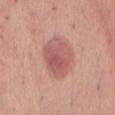Part of a total-body skin-imaging series; this lesion was reviewed on a skin check and was not flagged for biopsy.
The lesion is located on the front of the torso.
This is a white-light tile.
A male patient aged approximately 35.
Approximately 5 mm at its widest.
The lesion-visualizer software estimated an area of roughly 15 mm², an outline eccentricity of about 0.7 (0 = round, 1 = elongated), and two-axis asymmetry of about 0.1. And it measured a lesion color around L≈57 a*≈27 b*≈24 in CIELAB and a normalized lesion–skin contrast near 7. The analysis additionally found a border-irregularity index near 1/10, a within-lesion color-variation index near 4.5/10, and peripheral color asymmetry of about 1.5. The software also gave a classifier nevus-likeness of about 90/100 and a detector confidence of about 100 out of 100 that the crop contains a lesion.
A roughly 15 mm field-of-view crop from a total-body skin photograph.